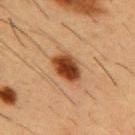Clinical impression: No biopsy was performed on this lesion — it was imaged during a full skin examination and was not determined to be concerning. Context: From the chest. Longest diameter approximately 4 mm. This image is a 15 mm lesion crop taken from a total-body photograph. The subject is a male aged approximately 55.